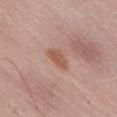Q: Was a biopsy performed?
A: imaged on a skin check; not biopsied
Q: Patient demographics?
A: male, aged 53–57
Q: How was this image acquired?
A: total-body-photography crop, ~15 mm field of view
Q: What lighting was used for the tile?
A: white-light illumination